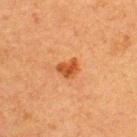The lesion was photographed on a routine skin check and not biopsied; there is no pathology result.
A male patient aged 38–42.
Imaged with cross-polarized lighting.
Cropped from a total-body skin-imaging series; the visible field is about 15 mm.
The lesion is on the upper back.